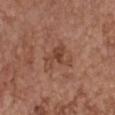workup: imaged on a skin check; not biopsied | location: the chest | patient: female, in their mid- to late 60s | acquisition: ~15 mm crop, total-body skin-cancer survey.